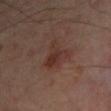Part of a total-body skin-imaging series; this lesion was reviewed on a skin check and was not flagged for biopsy. A male patient aged around 50. Longest diameter approximately 4 mm. Automated tile analysis of the lesion measured a footprint of about 8 mm², a shape eccentricity near 0.75, and two-axis asymmetry of about 0.45. It also reported a lesion color around L≈31 a*≈19 b*≈22 in CIELAB and roughly 7 lightness units darker than nearby skin. It also reported border irregularity of about 5 on a 0–10 scale and internal color variation of about 4.5 on a 0–10 scale. The software also gave an automated nevus-likeness rating near 55 out of 100 and a detector confidence of about 100 out of 100 that the crop contains a lesion. From the left arm. Captured under cross-polarized illumination. Cropped from a total-body skin-imaging series; the visible field is about 15 mm.